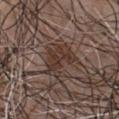notes: imaged on a skin check; not biopsied | acquisition: ~15 mm tile from a whole-body skin photo | automated lesion analysis: a mean CIELAB color near L≈34 a*≈15 b*≈22, a lesion–skin lightness drop of about 8, and a lesion-to-skin contrast of about 8 (normalized; higher = more distinct); lesion-presence confidence of about 95/100 | site: the chest | lesion size: ~4 mm (longest diameter) | subject: male, aged around 50.